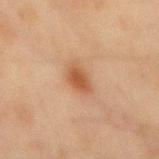notes=total-body-photography surveillance lesion; no biopsy | subject=male, about 45 years old | location=the back | image=total-body-photography crop, ~15 mm field of view.